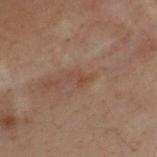An algorithmic analysis of the crop reported a lesion area of about 3 mm², an eccentricity of roughly 0.8, and two-axis asymmetry of about 0.4. It also reported an automated nevus-likeness rating near 0 out of 100. The tile uses cross-polarized illumination. From the chest. A close-up tile cropped from a whole-body skin photograph, about 15 mm across. A male subject, roughly 30 years of age.